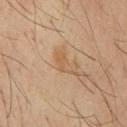patient — male, aged 58 to 62; image source — total-body-photography crop, ~15 mm field of view; tile lighting — cross-polarized; lesion diameter — ~3.5 mm (longest diameter); body site — the chest; TBP lesion metrics — an average lesion color of about L≈53 a*≈17 b*≈33 (CIELAB) and a lesion–skin lightness drop of about 6.